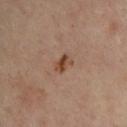Clinical summary: A male subject, about 45 years old. Cropped from a total-body skin-imaging series; the visible field is about 15 mm. The lesion is located on the chest.